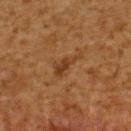Recorded during total-body skin imaging; not selected for excision or biopsy. The lesion is on the upper back. About 4 mm across. A close-up tile cropped from a whole-body skin photograph, about 15 mm across. The tile uses cross-polarized illumination. A male subject in their 60s.